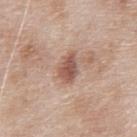The lesion was photographed on a routine skin check and not biopsied; there is no pathology result. Approximately 3.5 mm at its widest. Automated image analysis of the tile measured an outline eccentricity of about 0.8 (0 = round, 1 = elongated). The software also gave a border-irregularity rating of about 3/10 and radial color variation of about 1.5. The analysis additionally found a nevus-likeness score of about 80/100 and a lesion-detection confidence of about 100/100. The lesion is located on the chest. A male subject in their 80s. A lesion tile, about 15 mm wide, cut from a 3D total-body photograph.